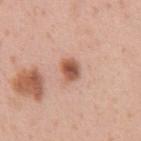<record>
  <biopsy_status>not biopsied; imaged during a skin examination</biopsy_status>
  <automated_metrics>
    <eccentricity>0.6</eccentricity>
    <shape_asymmetry>0.25</shape_asymmetry>
  </automated_metrics>
  <image>
    <source>total-body photography crop</source>
    <field_of_view_mm>15</field_of_view_mm>
  </image>
  <patient>
    <sex>male</sex>
    <age_approx>55</age_approx>
  </patient>
  <lighting>white-light</lighting>
  <site>abdomen</site>
</record>